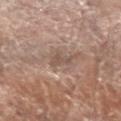Q: Is there a histopathology result?
A: imaged on a skin check; not biopsied
Q: Lesion location?
A: the left forearm
Q: Lesion size?
A: ≈2.5 mm
Q: What kind of image is this?
A: total-body-photography crop, ~15 mm field of view
Q: Who is the patient?
A: female, about 75 years old